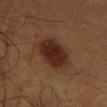notes=catalogued during a skin exam; not biopsied | lighting=cross-polarized | TBP lesion metrics=a border-irregularity index near 2/10, a color-variation rating of about 3.5/10, and radial color variation of about 1 | patient=male, aged 38–42 | body site=the chest | image=~15 mm tile from a whole-body skin photo.